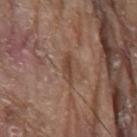biopsy_status: not biopsied; imaged during a skin examination
lighting: white-light
image:
  source: total-body photography crop
  field_of_view_mm: 15
automated_metrics:
  area_mm2_approx: 3.5
  eccentricity: 0.95
  shape_asymmetry: 0.25
  border_irregularity_0_10: 3.0
  peripheral_color_asymmetry: 0.5
  nevus_likeness_0_100: 5
  lesion_detection_confidence_0_100: 95
patient:
  sex: male
  age_approx: 80
lesion_size:
  long_diameter_mm_approx: 3.5
site: chest